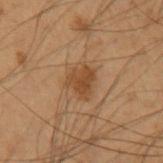biopsy_status: not biopsied; imaged during a skin examination
site: left upper arm
patient:
  sex: male
  age_approx: 55
image:
  source: total-body photography crop
  field_of_view_mm: 15